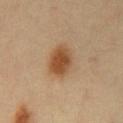Assessment: The lesion was photographed on a routine skin check and not biopsied; there is no pathology result. Clinical summary: Imaged with cross-polarized lighting. On the chest. A male subject, roughly 40 years of age. A close-up tile cropped from a whole-body skin photograph, about 15 mm across. About 4.5 mm across.